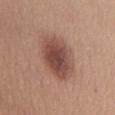Assessment: The lesion was tiled from a total-body skin photograph and was not biopsied. Context: From the front of the torso. A roughly 15 mm field-of-view crop from a total-body skin photograph. The tile uses white-light illumination. The subject is a female in their mid- to late 40s.